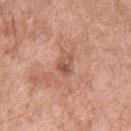The lesion was photographed on a routine skin check and not biopsied; there is no pathology result. Automated image analysis of the tile measured a mean CIELAB color near L≈54 a*≈24 b*≈29, roughly 10 lightness units darker than nearby skin, and a normalized border contrast of about 6.5. The analysis additionally found a nevus-likeness score of about 0/100 and a lesion-detection confidence of about 100/100. The lesion is on the upper back. Cropped from a total-body skin-imaging series; the visible field is about 15 mm. Longest diameter approximately 2.5 mm. A male subject, aged 48 to 52. Captured under white-light illumination.